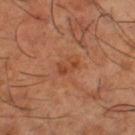Q: Was a biopsy performed?
A: catalogued during a skin exam; not biopsied
Q: Illumination type?
A: cross-polarized illumination
Q: What kind of image is this?
A: ~15 mm crop, total-body skin-cancer survey
Q: What are the patient's age and sex?
A: male, aged 63 to 67
Q: Where on the body is the lesion?
A: the leg
Q: Lesion size?
A: about 2.5 mm